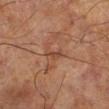Assessment:
Part of a total-body skin-imaging series; this lesion was reviewed on a skin check and was not flagged for biopsy.
Acquisition and patient details:
Imaged with cross-polarized lighting. This image is a 15 mm lesion crop taken from a total-body photograph. The recorded lesion diameter is about 2.5 mm. The lesion-visualizer software estimated a lesion area of about 2.5 mm², an eccentricity of roughly 0.9, and a symmetry-axis asymmetry near 0.35. The software also gave a border-irregularity rating of about 3.5/10, a color-variation rating of about 0.5/10, and radial color variation of about 0.5. The lesion is on the right lower leg. The patient is a male about 70 years old.